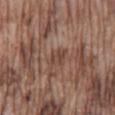Acquisition and patient details:
The lesion is located on the mid back. A 15 mm close-up tile from a total-body photography series done for melanoma screening. A male patient aged 73–77. Longest diameter approximately 2.5 mm. This is a white-light tile.